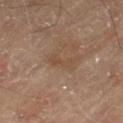{"biopsy_status": "not biopsied; imaged during a skin examination", "site": "left thigh", "image": {"source": "total-body photography crop", "field_of_view_mm": 15}, "lighting": "cross-polarized", "patient": {"sex": "male", "age_approx": 70}}